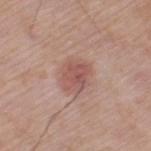workup: catalogued during a skin exam; not biopsied | anatomic site: the left thigh | patient: male, aged around 80 | lesion diameter: about 3.5 mm | illumination: white-light | image: 15 mm crop, total-body photography.